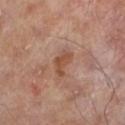biopsy status — imaged on a skin check; not biopsied | location — the leg | lighting — cross-polarized illumination | subject — male, aged 63 to 67 | diameter — about 3 mm | imaging modality — 15 mm crop, total-body photography.